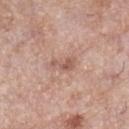Assessment: This lesion was catalogued during total-body skin photography and was not selected for biopsy. Clinical summary: This image is a 15 mm lesion crop taken from a total-body photograph. A female patient aged around 70. The lesion is on the right lower leg.